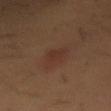Findings:
* follow-up · total-body-photography surveillance lesion; no biopsy
* imaging modality · ~15 mm crop, total-body skin-cancer survey
* patient · male, roughly 60 years of age
* tile lighting · cross-polarized
* size · about 2.5 mm
* site · the front of the torso